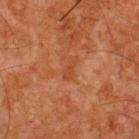The lesion was photographed on a routine skin check and not biopsied; there is no pathology result. The recorded lesion diameter is about 3 mm. Captured under cross-polarized illumination. A roughly 15 mm field-of-view crop from a total-body skin photograph. A male patient, roughly 65 years of age. From the upper back.